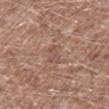The patient is a male aged around 60. Captured under white-light illumination. From the right lower leg. A 15 mm close-up extracted from a 3D total-body photography capture.